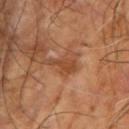follow-up: imaged on a skin check; not biopsied
subject: male, aged 63 to 67
imaging modality: total-body-photography crop, ~15 mm field of view
TBP lesion metrics: a lesion area of about 4.5 mm², an eccentricity of roughly 0.85, and two-axis asymmetry of about 0.5; a lesion color around L≈46 a*≈25 b*≈35 in CIELAB and a normalized lesion–skin contrast near 7; border irregularity of about 5.5 on a 0–10 scale
lesion diameter: ≈3.5 mm
anatomic site: the right upper arm